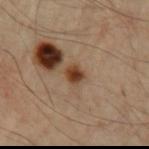The lesion was tiled from a total-body skin photograph and was not biopsied.
A roughly 15 mm field-of-view crop from a total-body skin photograph.
About 2.5 mm across.
A male patient, aged 63 to 67.
The tile uses cross-polarized illumination.
The lesion is located on the arm.